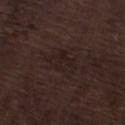Recorded during total-body skin imaging; not selected for excision or biopsy. A 15 mm close-up tile from a total-body photography series done for melanoma screening. Captured under white-light illumination. Longest diameter approximately 4 mm. From the leg. A male patient, approximately 70 years of age.